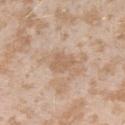This lesion was catalogued during total-body skin photography and was not selected for biopsy. Automated tile analysis of the lesion measured a footprint of about 5 mm², an eccentricity of roughly 0.7, and a symmetry-axis asymmetry near 0.25. And it measured roughly 7 lightness units darker than nearby skin and a lesion-to-skin contrast of about 5.5 (normalized; higher = more distinct). Measured at roughly 3 mm in maximum diameter. Cropped from a whole-body photographic skin survey; the tile spans about 15 mm. A female patient aged 23 to 27. The lesion is located on the left upper arm. This is a white-light tile.